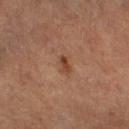Clinical impression: Part of a total-body skin-imaging series; this lesion was reviewed on a skin check and was not flagged for biopsy. Acquisition and patient details: The lesion is on the right thigh. A 15 mm close-up tile from a total-body photography series done for melanoma screening. A male patient, roughly 85 years of age or older. An algorithmic analysis of the crop reported two-axis asymmetry of about 0.3. And it measured an average lesion color of about L≈42 a*≈22 b*≈31 (CIELAB), roughly 9 lightness units darker than nearby skin, and a lesion-to-skin contrast of about 7.5 (normalized; higher = more distinct). Captured under cross-polarized illumination. Measured at roughly 2.5 mm in maximum diameter.